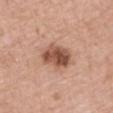{
  "biopsy_status": "not biopsied; imaged during a skin examination",
  "image": {
    "source": "total-body photography crop",
    "field_of_view_mm": 15
  },
  "patient": {
    "sex": "male",
    "age_approx": 75
  },
  "site": "left upper arm"
}